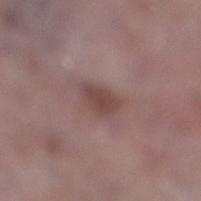Case summary:
* notes — no biopsy performed (imaged during a skin exam)
* lesion size — ≈3.5 mm
* site — the right lower leg
* subject — male, about 75 years old
* automated metrics — an average lesion color of about L≈45 a*≈18 b*≈20 (CIELAB), roughly 9 lightness units darker than nearby skin, and a normalized border contrast of about 7.5; an automated nevus-likeness rating near 0 out of 100
* illumination — white-light illumination
* image source — 15 mm crop, total-body photography